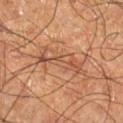notes: catalogued during a skin exam; not biopsied | lesion diameter: ~7 mm (longest diameter) | lighting: cross-polarized | body site: the right lower leg | acquisition: 15 mm crop, total-body photography | patient: male, aged 58–62.